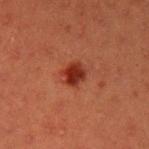Part of a total-body skin-imaging series; this lesion was reviewed on a skin check and was not flagged for biopsy.
Imaged with cross-polarized lighting.
The lesion is on the left upper arm.
A male patient, approximately 40 years of age.
Cropped from a total-body skin-imaging series; the visible field is about 15 mm.